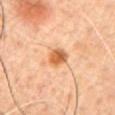* workup: catalogued during a skin exam; not biopsied
* patient: male, in their mid-40s
* TBP lesion metrics: an area of roughly 5.5 mm², an outline eccentricity of about 0.6 (0 = round, 1 = elongated), and a shape-asymmetry score of about 0.2 (0 = symmetric); an average lesion color of about L≈60 a*≈24 b*≈41 (CIELAB), about 13 CIELAB-L* units darker than the surrounding skin, and a normalized border contrast of about 9; a color-variation rating of about 4.5/10 and a peripheral color-asymmetry measure near 1.5; a classifier nevus-likeness of about 100/100 and a detector confidence of about 100 out of 100 that the crop contains a lesion
* site: the chest
* lesion diameter: ≈3 mm
* image source: total-body-photography crop, ~15 mm field of view
* tile lighting: cross-polarized illumination